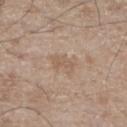The lesion was tiled from a total-body skin photograph and was not biopsied. The recorded lesion diameter is about 3 mm. Captured under white-light illumination. From the right lower leg. A male subject, roughly 65 years of age. A 15 mm close-up extracted from a 3D total-body photography capture.